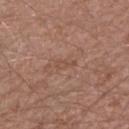Q: Was a biopsy performed?
A: total-body-photography surveillance lesion; no biopsy
Q: How was this image acquired?
A: 15 mm crop, total-body photography
Q: What is the lesion's diameter?
A: ≈2.5 mm
Q: What is the anatomic site?
A: the right upper arm
Q: Patient demographics?
A: male, approximately 60 years of age
Q: What did automated image analysis measure?
A: a lesion area of about 2.5 mm² and a symmetry-axis asymmetry near 0.4; internal color variation of about 0 on a 0–10 scale and peripheral color asymmetry of about 0